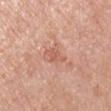{"biopsy_status": "not biopsied; imaged during a skin examination", "site": "arm", "patient": {"sex": "male", "age_approx": 55}, "lighting": "white-light", "lesion_size": {"long_diameter_mm_approx": 2.5}, "image": {"source": "total-body photography crop", "field_of_view_mm": 15}}